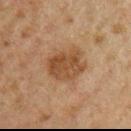About 5 mm across. Imaged with cross-polarized lighting. The subject is a male roughly 50 years of age. From the right upper arm. A region of skin cropped from a whole-body photographic capture, roughly 15 mm wide.